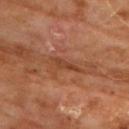Findings:
* follow-up · total-body-photography surveillance lesion; no biopsy
* image · 15 mm crop, total-body photography
* site · the chest
* image-analysis metrics · an average lesion color of about L≈40 a*≈24 b*≈32 (CIELAB) and about 7 CIELAB-L* units darker than the surrounding skin
* patient · male, aged 58 to 62
* tile lighting · cross-polarized illumination
* lesion size · ~3 mm (longest diameter)